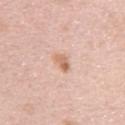biopsy_status: not biopsied; imaged during a skin examination
patient:
  sex: female
  age_approx: 40
lighting: white-light
image:
  source: total-body photography crop
  field_of_view_mm: 15
site: left arm
automated_metrics:
  cielab_L: 66
  cielab_a: 21
  cielab_b: 32
  vs_skin_contrast_norm: 7.0
  border_irregularity_0_10: 2.5
  peripheral_color_asymmetry: 2.0
lesion_size:
  long_diameter_mm_approx: 2.5